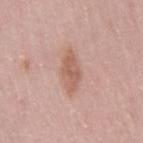No biopsy was performed on this lesion — it was imaged during a full skin examination and was not determined to be concerning. A female patient, aged 28 to 32. The lesion is on the right thigh. This is a white-light tile. The total-body-photography lesion software estimated a lesion area of about 7 mm², an outline eccentricity of about 0.9 (0 = round, 1 = elongated), and two-axis asymmetry of about 0.3. And it measured a lesion-detection confidence of about 100/100. The lesion's longest dimension is about 4.5 mm. A region of skin cropped from a whole-body photographic capture, roughly 15 mm wide.